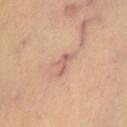Clinical impression:
The lesion was photographed on a routine skin check and not biopsied; there is no pathology result.
Acquisition and patient details:
A roughly 15 mm field-of-view crop from a total-body skin photograph. On the chest. Measured at roughly 3 mm in maximum diameter.